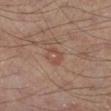The lesion was tiled from a total-body skin photograph and was not biopsied. Cropped from a whole-body photographic skin survey; the tile spans about 15 mm. An algorithmic analysis of the crop reported a lesion area of about 3 mm², an eccentricity of roughly 0.8, and a symmetry-axis asymmetry near 0.7. The analysis additionally found a border-irregularity index near 7/10 and a peripheral color-asymmetry measure near 0. And it measured a nevus-likeness score of about 0/100 and lesion-presence confidence of about 100/100. The tile uses cross-polarized illumination. Approximately 2.5 mm at its widest. A male subject aged approximately 55. The lesion is on the left lower leg.